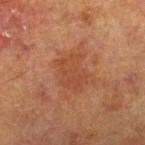{
  "biopsy_status": "not biopsied; imaged during a skin examination",
  "lighting": "cross-polarized",
  "lesion_size": {
    "long_diameter_mm_approx": 4.0
  },
  "site": "right lower leg",
  "patient": {
    "sex": "male",
    "age_approx": 70
  },
  "automated_metrics": {
    "area_mm2_approx": 10.0,
    "eccentricity": 0.65,
    "vs_skin_contrast_norm": 5.0,
    "border_irregularity_0_10": 3.5,
    "color_variation_0_10": 2.0,
    "nevus_likeness_0_100": 0,
    "lesion_detection_confidence_0_100": 100
  },
  "image": {
    "source": "total-body photography crop",
    "field_of_view_mm": 15
  }
}